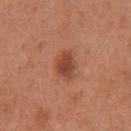This lesion was catalogued during total-body skin photography and was not selected for biopsy.